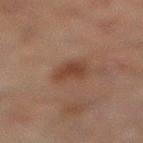biopsy_status: not biopsied; imaged during a skin examination
lesion_size:
  long_diameter_mm_approx: 3.0
patient:
  sex: male
  age_approx: 60
site: left leg
image:
  source: total-body photography crop
  field_of_view_mm: 15
automated_metrics:
  vs_skin_contrast_norm: 7.5
  nevus_likeness_0_100: 55